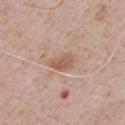{"biopsy_status": "not biopsied; imaged during a skin examination", "lesion_size": {"long_diameter_mm_approx": 3.0}, "lighting": "white-light", "image": {"source": "total-body photography crop", "field_of_view_mm": 15}, "patient": {"sex": "male", "age_approx": 55}}